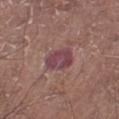follow-up = total-body-photography surveillance lesion; no biopsy | tile lighting = white-light | lesion diameter = ~4 mm (longest diameter) | anatomic site = the left lower leg | acquisition = ~15 mm crop, total-body skin-cancer survey | subject = male, in their 70s.